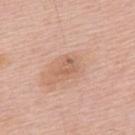Q: Was a biopsy performed?
A: no biopsy performed (imaged during a skin exam)
Q: What did automated image analysis measure?
A: a lesion area of about 3.5 mm² and a symmetry-axis asymmetry near 0.45; an average lesion color of about L≈60 a*≈21 b*≈31 (CIELAB) and a lesion-to-skin contrast of about 5.5 (normalized; higher = more distinct); a border-irregularity rating of about 4.5/10, internal color variation of about 3 on a 0–10 scale, and radial color variation of about 1; a nevus-likeness score of about 0/100
Q: What is the lesion's diameter?
A: about 2.5 mm
Q: Illumination type?
A: white-light illumination
Q: What are the patient's age and sex?
A: male, approximately 55 years of age
Q: What is the anatomic site?
A: the upper back
Q: What kind of image is this?
A: 15 mm crop, total-body photography